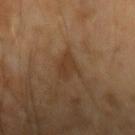lesion size: about 3.5 mm | subject: male, aged 63 to 67 | TBP lesion metrics: a footprint of about 5 mm² and two-axis asymmetry of about 0.4 | acquisition: 15 mm crop, total-body photography.